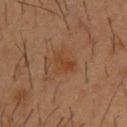A male subject, in their mid-50s. The lesion is located on the chest. A 15 mm crop from a total-body photograph taken for skin-cancer surveillance.